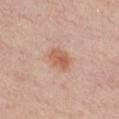Clinical impression: This lesion was catalogued during total-body skin photography and was not selected for biopsy.